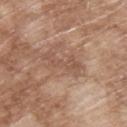No biopsy was performed on this lesion — it was imaged during a full skin examination and was not determined to be concerning. Measured at roughly 5 mm in maximum diameter. A 15 mm crop from a total-body photograph taken for skin-cancer surveillance. A male patient, roughly 65 years of age. The lesion is located on the upper back.